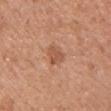Q: Is there a histopathology result?
A: imaged on a skin check; not biopsied
Q: What is the imaging modality?
A: ~15 mm tile from a whole-body skin photo
Q: How large is the lesion?
A: about 2.5 mm
Q: What did automated image analysis measure?
A: a lesion area of about 4.5 mm² and an eccentricity of roughly 0.65; a border-irregularity index near 2/10, a color-variation rating of about 1.5/10, and radial color variation of about 0.5; an automated nevus-likeness rating near 15 out of 100
Q: Patient demographics?
A: female, roughly 35 years of age
Q: Lesion location?
A: the chest
Q: What lighting was used for the tile?
A: white-light illumination